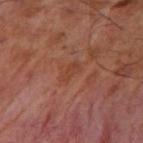The lesion was photographed on a routine skin check and not biopsied; there is no pathology result. This is a cross-polarized tile. A male patient about 55 years old. The recorded lesion diameter is about 2.5 mm. A 15 mm close-up extracted from a 3D total-body photography capture. Located on the right upper arm.